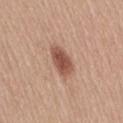{"biopsy_status": "not biopsied; imaged during a skin examination", "lighting": "white-light", "lesion_size": {"long_diameter_mm_approx": 4.0}, "image": {"source": "total-body photography crop", "field_of_view_mm": 15}, "site": "lower back", "patient": {"sex": "female", "age_approx": 55}}